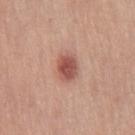Assessment: The lesion was tiled from a total-body skin photograph and was not biopsied. Acquisition and patient details: The patient is a female roughly 50 years of age. The recorded lesion diameter is about 3.5 mm. Captured under white-light illumination. The lesion is on the right thigh. A 15 mm close-up tile from a total-body photography series done for melanoma screening.